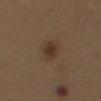Q: Was this lesion biopsied?
A: catalogued during a skin exam; not biopsied
Q: What kind of image is this?
A: 15 mm crop, total-body photography
Q: What are the patient's age and sex?
A: female, aged 38 to 42
Q: Illumination type?
A: white-light illumination
Q: How large is the lesion?
A: ≈2.5 mm
Q: Where on the body is the lesion?
A: the front of the torso
Q: Automated lesion metrics?
A: a footprint of about 5 mm², an eccentricity of roughly 0.6, and a symmetry-axis asymmetry near 0.2; a mean CIELAB color near L≈33 a*≈16 b*≈24 and roughly 8 lightness units darker than nearby skin; a classifier nevus-likeness of about 95/100 and a lesion-detection confidence of about 100/100